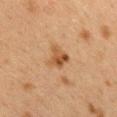{"biopsy_status": "not biopsied; imaged during a skin examination", "site": "upper back", "patient": {"sex": "female", "age_approx": 40}, "image": {"source": "total-body photography crop", "field_of_view_mm": 15}}